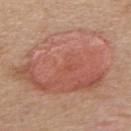  site: upper back
  image:
    source: total-body photography crop
    field_of_view_mm: 15
  lighting: white-light
  patient:
    sex: female
    age_approx: 40